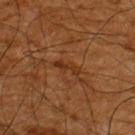Recorded during total-body skin imaging; not selected for excision or biopsy. Captured under cross-polarized illumination. The lesion's longest dimension is about 3 mm. An algorithmic analysis of the crop reported a border-irregularity rating of about 4.5/10. The analysis additionally found a nevus-likeness score of about 0/100 and a lesion-detection confidence of about 80/100. Located on the upper back. Cropped from a whole-body photographic skin survey; the tile spans about 15 mm. A male subject aged 63–67.